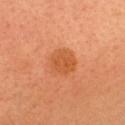* follow-up: no biopsy performed (imaged during a skin exam)
* lesion diameter: ~3.5 mm (longest diameter)
* automated lesion analysis: a border-irregularity rating of about 1/10, a color-variation rating of about 2.5/10, and a peripheral color-asymmetry measure near 1; a nevus-likeness score of about 60/100 and a lesion-detection confidence of about 100/100
* patient: female, aged 28 to 32
* image: ~15 mm tile from a whole-body skin photo
* site: the head or neck
* tile lighting: cross-polarized